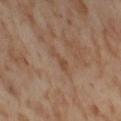Part of a total-body skin-imaging series; this lesion was reviewed on a skin check and was not flagged for biopsy. A 15 mm close-up extracted from a 3D total-body photography capture. A female patient, in their mid-50s. This is a cross-polarized tile. The recorded lesion diameter is about 3 mm. Automated image analysis of the tile measured internal color variation of about 0.5 on a 0–10 scale and radial color variation of about 0. Located on the right thigh.